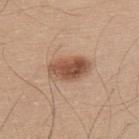Captured during whole-body skin photography for melanoma surveillance; the lesion was not biopsied.
The subject is a male aged around 45.
This is a white-light tile.
A 15 mm close-up tile from a total-body photography series done for melanoma screening.
Located on the upper back.
The recorded lesion diameter is about 5 mm.
Automated tile analysis of the lesion measured a lesion area of about 11 mm² and a shape-asymmetry score of about 0.15 (0 = symmetric). It also reported a mean CIELAB color near L≈53 a*≈20 b*≈31, about 14 CIELAB-L* units darker than the surrounding skin, and a normalized lesion–skin contrast near 9.5. It also reported a within-lesion color-variation index near 6/10 and a peripheral color-asymmetry measure near 2.